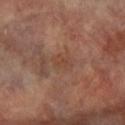| field | value |
|---|---|
| workup | total-body-photography surveillance lesion; no biopsy |
| acquisition | 15 mm crop, total-body photography |
| TBP lesion metrics | a lesion area of about 3.5 mm² and two-axis asymmetry of about 0.35; a mean CIELAB color near L≈42 a*≈19 b*≈28 and a lesion-to-skin contrast of about 5 (normalized; higher = more distinct); a nevus-likeness score of about 0/100 |
| lesion size | ~2.5 mm (longest diameter) |
| body site | the left arm |
| lighting | cross-polarized illumination |
| subject | female, aged approximately 65 |